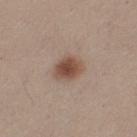Impression: The lesion was tiled from a total-body skin photograph and was not biopsied. Image and clinical context: A female patient, aged 23–27. A roughly 15 mm field-of-view crop from a total-body skin photograph. Automated image analysis of the tile measured an area of roughly 7.5 mm², an eccentricity of roughly 0.6, and two-axis asymmetry of about 0.2. The software also gave a lesion color around L≈49 a*≈18 b*≈26 in CIELAB and about 12 CIELAB-L* units darker than the surrounding skin. The software also gave a nevus-likeness score of about 100/100 and a lesion-detection confidence of about 100/100. The lesion is located on the left thigh. Captured under white-light illumination. Approximately 3 mm at its widest.